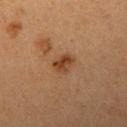Recorded during total-body skin imaging; not selected for excision or biopsy. This image is a 15 mm lesion crop taken from a total-body photograph. A female subject, in their 40s. About 2.5 mm across. Located on the right upper arm.